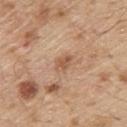Clinical summary:
The recorded lesion diameter is about 2.5 mm. From the upper back. The patient is a male aged approximately 70. This image is a 15 mm lesion crop taken from a total-body photograph.